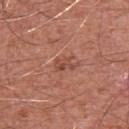Impression:
Recorded during total-body skin imaging; not selected for excision or biopsy.
Clinical summary:
This is a white-light tile. A region of skin cropped from a whole-body photographic capture, roughly 15 mm wide. The patient is a male aged approximately 80. Located on the arm. Measured at roughly 3 mm in maximum diameter. The total-body-photography lesion software estimated an average lesion color of about L≈48 a*≈26 b*≈28 (CIELAB). The analysis additionally found border irregularity of about 4 on a 0–10 scale and peripheral color asymmetry of about 1. And it measured a classifier nevus-likeness of about 5/100 and lesion-presence confidence of about 100/100.